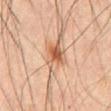Clinical impression: The lesion was tiled from a total-body skin photograph and was not biopsied. Context: This is a cross-polarized tile. A male patient, about 70 years old. The lesion is located on the back. Longest diameter approximately 3.5 mm. Cropped from a whole-body photographic skin survey; the tile spans about 15 mm. The lesion-visualizer software estimated a lesion area of about 6.5 mm², an outline eccentricity of about 0.55 (0 = round, 1 = elongated), and two-axis asymmetry of about 0.35. It also reported an average lesion color of about L≈48 a*≈19 b*≈30 (CIELAB) and a normalized border contrast of about 8. It also reported a nevus-likeness score of about 90/100 and a lesion-detection confidence of about 100/100.